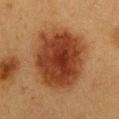Clinical impression:
No biopsy was performed on this lesion — it was imaged during a full skin examination and was not determined to be concerning.
Clinical summary:
This is a cross-polarized tile. An algorithmic analysis of the crop reported a border-irregularity rating of about 1.5/10, a color-variation rating of about 5/10, and radial color variation of about 1.5. The lesion is located on the chest. A 15 mm close-up tile from a total-body photography series done for melanoma screening. The subject is a female aged approximately 40. Approximately 8 mm at its widest.